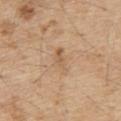Q: Was this lesion biopsied?
A: catalogued during a skin exam; not biopsied
Q: What lighting was used for the tile?
A: white-light
Q: How was this image acquired?
A: ~15 mm crop, total-body skin-cancer survey
Q: How large is the lesion?
A: about 3 mm
Q: What is the anatomic site?
A: the upper back
Q: What are the patient's age and sex?
A: male, aged 68–72